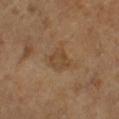The lesion was photographed on a routine skin check and not biopsied; there is no pathology result.